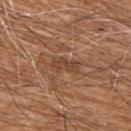Assessment:
Imaged during a routine full-body skin examination; the lesion was not biopsied and no histopathology is available.
Context:
A 15 mm crop from a total-body photograph taken for skin-cancer surveillance. A male patient, about 75 years old. Captured under white-light illumination. The lesion's longest dimension is about 3.5 mm. Located on the right upper arm.